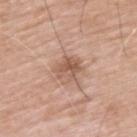Q: Is there a histopathology result?
A: no biopsy performed (imaged during a skin exam)
Q: What is the lesion's diameter?
A: ~3 mm (longest diameter)
Q: What are the patient's age and sex?
A: male, aged around 65
Q: Where on the body is the lesion?
A: the upper back
Q: What kind of image is this?
A: ~15 mm crop, total-body skin-cancer survey
Q: Illumination type?
A: white-light illumination